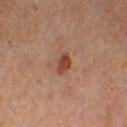Clinical impression:
Captured during whole-body skin photography for melanoma surveillance; the lesion was not biopsied.
Acquisition and patient details:
A region of skin cropped from a whole-body photographic capture, roughly 15 mm wide. Located on the right thigh. The subject is a female aged 48–52.